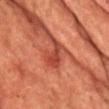{"biopsy_status": "not biopsied; imaged during a skin examination", "site": "chest", "image": {"source": "total-body photography crop", "field_of_view_mm": 15}, "lighting": "cross-polarized", "patient": {"sex": "male", "age_approx": 65}}